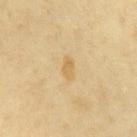Findings:
- workup: catalogued during a skin exam; not biopsied